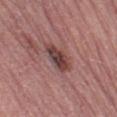Findings:
- workup — imaged on a skin check; not biopsied
- automated lesion analysis — a border-irregularity index near 2.5/10 and a peripheral color-asymmetry measure near 2; an automated nevus-likeness rating near 10 out of 100 and a detector confidence of about 100 out of 100 that the crop contains a lesion
- body site — the left thigh
- subject — female, in their mid-60s
- image — 15 mm crop, total-body photography
- tile lighting — white-light